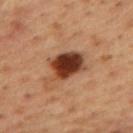| feature | finding |
|---|---|
| notes | no biopsy performed (imaged during a skin exam) |
| image-analysis metrics | a footprint of about 13 mm², a shape eccentricity near 0.75, and a shape-asymmetry score of about 0.3 (0 = symmetric); a normalized border contrast of about 13.5; a classifier nevus-likeness of about 95/100 |
| location | the upper back |
| image source | 15 mm crop, total-body photography |
| illumination | cross-polarized illumination |
| diameter | ≈5.5 mm |
| patient | male, aged approximately 55 |